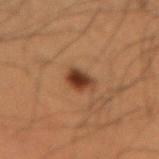<tbp_lesion>
  <biopsy_status>not biopsied; imaged during a skin examination</biopsy_status>
  <site>right upper arm</site>
  <patient>
    <sex>male</sex>
    <age_approx>50</age_approx>
  </patient>
  <image>
    <source>total-body photography crop</source>
    <field_of_view_mm>15</field_of_view_mm>
  </image>
  <lesion_size>
    <long_diameter_mm_approx>2.5</long_diameter_mm_approx>
  </lesion_size>
  <lighting>cross-polarized</lighting>
</tbp_lesion>